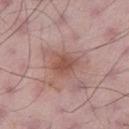The lesion was photographed on a routine skin check and not biopsied; there is no pathology result.